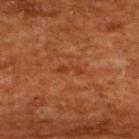Part of a total-body skin-imaging series; this lesion was reviewed on a skin check and was not flagged for biopsy. The total-body-photography lesion software estimated a lesion area of about 3 mm², an outline eccentricity of about 0.95 (0 = round, 1 = elongated), and two-axis asymmetry of about 0.5. And it measured a lesion color around L≈38 a*≈28 b*≈36 in CIELAB, a lesion–skin lightness drop of about 6, and a normalized lesion–skin contrast near 5. The analysis additionally found a border-irregularity index near 6/10 and radial color variation of about 0. And it measured an automated nevus-likeness rating near 0 out of 100 and a detector confidence of about 100 out of 100 that the crop contains a lesion. Captured under cross-polarized illumination. The patient is a male aged 63–67. On the back. This image is a 15 mm lesion crop taken from a total-body photograph.